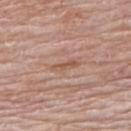Recorded during total-body skin imaging; not selected for excision or biopsy. The lesion's longest dimension is about 3.5 mm. The tile uses white-light illumination. This image is a 15 mm lesion crop taken from a total-body photograph. The lesion is located on the upper back. A female patient, in their mid-60s.